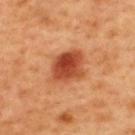Captured under cross-polarized illumination.
From the back.
A 15 mm close-up extracted from a 3D total-body photography capture.
Automated tile analysis of the lesion measured a footprint of about 13 mm², an outline eccentricity of about 0.65 (0 = round, 1 = elongated), and a shape-asymmetry score of about 0.2 (0 = symmetric). The software also gave about 15 CIELAB-L* units darker than the surrounding skin. And it measured a border-irregularity rating of about 2/10, a within-lesion color-variation index near 6/10, and a peripheral color-asymmetry measure near 2. It also reported an automated nevus-likeness rating near 100 out of 100 and lesion-presence confidence of about 100/100.
A male patient, in their 50s.
The recorded lesion diameter is about 4.5 mm.